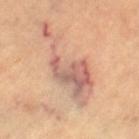Findings:
– workup · no biopsy performed (imaged during a skin exam)
– tile lighting · cross-polarized
– image · total-body-photography crop, ~15 mm field of view
– site · the left thigh
– size · about 10 mm
– image-analysis metrics · a border-irregularity rating of about 9.5/10, a color-variation rating of about 7.5/10, and radial color variation of about 2.5
– patient · aged around 60